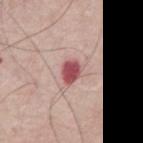A region of skin cropped from a whole-body photographic capture, roughly 15 mm wide.
A male patient, approximately 60 years of age.
Located on the chest.